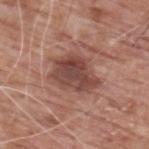Q: Is there a histopathology result?
A: catalogued during a skin exam; not biopsied
Q: Who is the patient?
A: male, aged around 60
Q: What did automated image analysis measure?
A: an average lesion color of about L≈45 a*≈22 b*≈24 (CIELAB), roughly 12 lightness units darker than nearby skin, and a normalized border contrast of about 9; an automated nevus-likeness rating near 0 out of 100 and a detector confidence of about 100 out of 100 that the crop contains a lesion
Q: How large is the lesion?
A: about 5.5 mm
Q: What is the anatomic site?
A: the upper back
Q: Illumination type?
A: white-light
Q: How was this image acquired?
A: ~15 mm crop, total-body skin-cancer survey